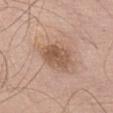Imaged during a routine full-body skin examination; the lesion was not biopsied and no histopathology is available. Measured at roughly 4 mm in maximum diameter. A lesion tile, about 15 mm wide, cut from a 3D total-body photograph. The patient is a male in their mid-70s. The lesion is located on the abdomen.